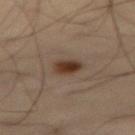Findings:
- workup: catalogued during a skin exam; not biopsied
- location: the abdomen
- diameter: ~2.5 mm (longest diameter)
- acquisition: ~15 mm crop, total-body skin-cancer survey
- subject: male, aged around 55
- illumination: cross-polarized